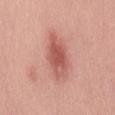Part of a total-body skin-imaging series; this lesion was reviewed on a skin check and was not flagged for biopsy.
The patient is a male in their 30s.
An algorithmic analysis of the crop reported a lesion area of about 12 mm², a shape eccentricity near 0.9, and two-axis asymmetry of about 0.2. The analysis additionally found a lesion color around L≈57 a*≈28 b*≈27 in CIELAB and a normalized lesion–skin contrast near 7.5. And it measured border irregularity of about 2.5 on a 0–10 scale, a color-variation rating of about 4.5/10, and radial color variation of about 1.5. And it measured an automated nevus-likeness rating near 85 out of 100 and a detector confidence of about 100 out of 100 that the crop contains a lesion.
The tile uses white-light illumination.
About 6 mm across.
A close-up tile cropped from a whole-body skin photograph, about 15 mm across.